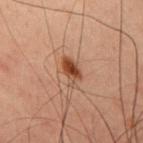Clinical summary:
The tile uses cross-polarized illumination. The total-body-photography lesion software estimated a lesion area of about 5.5 mm², a shape eccentricity near 0.8, and a symmetry-axis asymmetry near 0.35. It also reported a border-irregularity index near 3.5/10, a within-lesion color-variation index near 3.5/10, and peripheral color asymmetry of about 1. The patient is a male aged around 60. Located on the chest. Cropped from a total-body skin-imaging series; the visible field is about 15 mm. About 3.5 mm across.